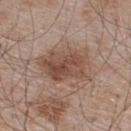| field | value |
|---|---|
| anatomic site | the upper back |
| subject | male, aged 53–57 |
| illumination | white-light illumination |
| image source | ~15 mm tile from a whole-body skin photo |